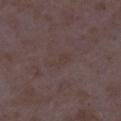{"biopsy_status": "not biopsied; imaged during a skin examination", "patient": {"sex": "female", "age_approx": 35}, "lighting": "white-light", "site": "right lower leg", "lesion_size": {"long_diameter_mm_approx": 3.0}, "image": {"source": "total-body photography crop", "field_of_view_mm": 15}, "automated_metrics": {"area_mm2_approx": 3.5, "eccentricity": 0.9, "shape_asymmetry": 0.45}}